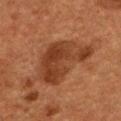<case>
<biopsy_status>not biopsied; imaged during a skin examination</biopsy_status>
<image>
  <source>total-body photography crop</source>
  <field_of_view_mm>15</field_of_view_mm>
</image>
<automated_metrics>
  <area_mm2_approx>22.0</area_mm2_approx>
  <eccentricity>0.65</eccentricity>
  <shape_asymmetry>0.4</shape_asymmetry>
</automated_metrics>
<patient>
  <sex>male</sex>
  <age_approx>60</age_approx>
</patient>
<site>head or neck</site>
</case>